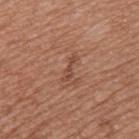notes: no biopsy performed (imaged during a skin exam) | lighting: white-light illumination | lesion size: ~3 mm (longest diameter) | acquisition: ~15 mm tile from a whole-body skin photo | location: the back | patient: male, aged 53–57.